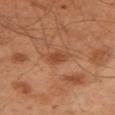No biopsy was performed on this lesion — it was imaged during a full skin examination and was not determined to be concerning. A male patient aged 48–52. A 15 mm close-up tile from a total-body photography series done for melanoma screening. This is a cross-polarized tile. Measured at roughly 3 mm in maximum diameter. The total-body-photography lesion software estimated a lesion area of about 4.5 mm² and an eccentricity of roughly 0.7. The analysis additionally found a nevus-likeness score of about 45/100 and a lesion-detection confidence of about 100/100. The lesion is located on the arm.